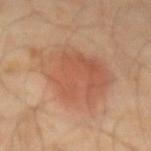notes: total-body-photography surveillance lesion; no biopsy | anatomic site: the right forearm | image: 15 mm crop, total-body photography | subject: male, roughly 45 years of age | diameter: about 8.5 mm | lighting: cross-polarized illumination.